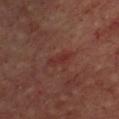Q: Is there a histopathology result?
A: no biopsy performed (imaged during a skin exam)
Q: How large is the lesion?
A: ≈3 mm
Q: How was this image acquired?
A: ~15 mm crop, total-body skin-cancer survey
Q: Where on the body is the lesion?
A: the front of the torso
Q: Automated lesion metrics?
A: a mean CIELAB color near L≈30 a*≈26 b*≈22
Q: How was the tile lit?
A: cross-polarized
Q: Patient demographics?
A: female, aged 43–47